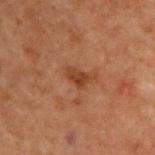This lesion was catalogued during total-body skin photography and was not selected for biopsy. The patient is a male about 70 years old. The recorded lesion diameter is about 3 mm. Automated image analysis of the tile measured a lesion color around L≈31 a*≈20 b*≈28 in CIELAB, roughly 7 lightness units darker than nearby skin, and a normalized border contrast of about 7.5. The software also gave a border-irregularity index near 2.5/10, a color-variation rating of about 1.5/10, and a peripheral color-asymmetry measure near 0.5. The analysis additionally found a lesion-detection confidence of about 100/100. A 15 mm close-up tile from a total-body photography series done for melanoma screening. Imaged with cross-polarized lighting. The lesion is located on the back.